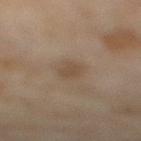<tbp_lesion>
  <biopsy_status>not biopsied; imaged during a skin examination</biopsy_status>
  <lighting>cross-polarized</lighting>
  <lesion_size>
    <long_diameter_mm_approx>3.0</long_diameter_mm_approx>
  </lesion_size>
  <image>
    <source>total-body photography crop</source>
    <field_of_view_mm>15</field_of_view_mm>
  </image>
  <patient>
    <sex>female</sex>
    <age_approx>60</age_approx>
  </patient>
  <site>right lower leg</site>
</tbp_lesion>